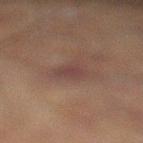Impression: Recorded during total-body skin imaging; not selected for excision or biopsy. Clinical summary: A 15 mm crop from a total-body photograph taken for skin-cancer surveillance. A male subject, aged 58–62. Located on the left lower leg.